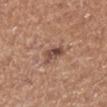  biopsy_status: not biopsied; imaged during a skin examination
  patient:
    sex: male
    age_approx: 75
  image:
    source: total-body photography crop
    field_of_view_mm: 15
  site: left lower leg